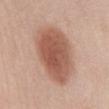Case summary:
- notes · no biopsy performed (imaged during a skin exam)
- lesion diameter · ≈8.5 mm
- illumination · white-light illumination
- anatomic site · the abdomen
- image · ~15 mm tile from a whole-body skin photo
- subject · female, in their 50s